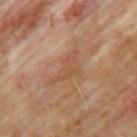Impression: Captured during whole-body skin photography for melanoma surveillance; the lesion was not biopsied. Clinical summary: A 15 mm close-up tile from a total-body photography series done for melanoma screening. The lesion-visualizer software estimated a footprint of about 6.5 mm², a shape eccentricity near 0.9, and a shape-asymmetry score of about 0.5 (0 = symmetric). The software also gave a mean CIELAB color near L≈49 a*≈20 b*≈30, about 7 CIELAB-L* units darker than the surrounding skin, and a normalized border contrast of about 5. The lesion's longest dimension is about 5 mm. The patient is a male in their mid- to late 60s. Imaged with cross-polarized lighting. Located on the arm.